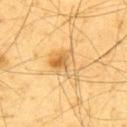site = the upper back | image-analysis metrics = a footprint of about 9.5 mm², an eccentricity of roughly 0.55, and a symmetry-axis asymmetry near 0.25; a lesion color around L≈70 a*≈19 b*≈48 in CIELAB; border irregularity of about 2.5 on a 0–10 scale and a peripheral color-asymmetry measure near 3; a classifier nevus-likeness of about 60/100 | lighting = cross-polarized illumination | subject = male, aged 63–67 | imaging modality = ~15 mm crop, total-body skin-cancer survey | lesion diameter = about 3.5 mm.